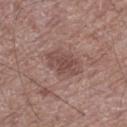Notes:
* notes: catalogued during a skin exam; not biopsied
* imaging modality: total-body-photography crop, ~15 mm field of view
* location: the leg
* size: about 4 mm
* subject: male, aged 68–72